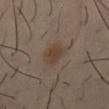This lesion was catalogued during total-body skin photography and was not selected for biopsy.
From the chest.
Imaged with cross-polarized lighting.
A 15 mm close-up extracted from a 3D total-body photography capture.
Measured at roughly 3.5 mm in maximum diameter.
The patient is a male about 40 years old.